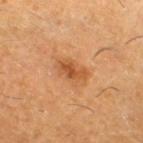The lesion was tiled from a total-body skin photograph and was not biopsied. A 15 mm crop from a total-body photograph taken for skin-cancer surveillance. Captured under cross-polarized illumination. A male subject, aged 58 to 62. Approximately 4 mm at its widest. The lesion-visualizer software estimated an average lesion color of about L≈51 a*≈25 b*≈39 (CIELAB). It also reported a border-irregularity rating of about 2.5/10 and radial color variation of about 1.5. The software also gave an automated nevus-likeness rating near 85 out of 100 and lesion-presence confidence of about 100/100. The lesion is located on the right thigh.